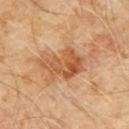The lesion was tiled from a total-body skin photograph and was not biopsied. Longest diameter approximately 5.5 mm. The lesion is located on the chest. A male patient aged 58 to 62. Automated tile analysis of the lesion measured an area of roughly 13 mm², an outline eccentricity of about 0.75 (0 = round, 1 = elongated), and a shape-asymmetry score of about 0.4 (0 = symmetric). And it measured a lesion color around L≈53 a*≈23 b*≈38 in CIELAB, a lesion–skin lightness drop of about 10, and a normalized lesion–skin contrast near 7.5. It also reported an automated nevus-likeness rating near 0 out of 100 and a lesion-detection confidence of about 100/100. A close-up tile cropped from a whole-body skin photograph, about 15 mm across.